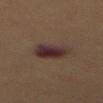Q: Was this lesion biopsied?
A: total-body-photography surveillance lesion; no biopsy
Q: What kind of image is this?
A: total-body-photography crop, ~15 mm field of view
Q: What are the patient's age and sex?
A: male, about 55 years old
Q: Lesion size?
A: ≈3.5 mm
Q: What did automated image analysis measure?
A: a footprint of about 9.5 mm² and an outline eccentricity of about 0.6 (0 = round, 1 = elongated); border irregularity of about 2 on a 0–10 scale and a peripheral color-asymmetry measure near 1.5
Q: What lighting was used for the tile?
A: cross-polarized
Q: Lesion location?
A: the mid back